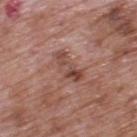No biopsy was performed on this lesion — it was imaged during a full skin examination and was not determined to be concerning. A region of skin cropped from a whole-body photographic capture, roughly 15 mm wide. From the back. A male subject, in their 70s. This is a white-light tile. The lesion's longest dimension is about 4.5 mm.